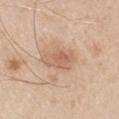Findings:
• workup — imaged on a skin check; not biopsied
• illumination — white-light illumination
• diameter — ~4 mm (longest diameter)
• site — the arm
• image — ~15 mm crop, total-body skin-cancer survey
• subject — male, roughly 65 years of age